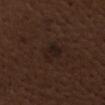follow-up = catalogued during a skin exam; not biopsied | anatomic site = the head or neck | subject = male, roughly 70 years of age | tile lighting = white-light illumination | acquisition = 15 mm crop, total-body photography | lesion size = ≈3 mm.